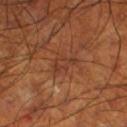Case summary:
- notes — imaged on a skin check; not biopsied
- body site — the leg
- tile lighting — cross-polarized illumination
- subject — male, about 70 years old
- acquisition — total-body-photography crop, ~15 mm field of view